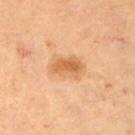Part of a total-body skin-imaging series; this lesion was reviewed on a skin check and was not flagged for biopsy.
Cropped from a whole-body photographic skin survey; the tile spans about 15 mm.
A female patient, in their mid-40s.
Located on the left upper arm.